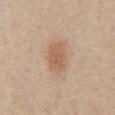Findings:
* biopsy status · total-body-photography surveillance lesion; no biopsy
* automated metrics · a lesion area of about 8.5 mm², an outline eccentricity of about 0.8 (0 = round, 1 = elongated), and two-axis asymmetry of about 0.2; a mean CIELAB color near L≈59 a*≈19 b*≈31, about 9 CIELAB-L* units darker than the surrounding skin, and a normalized lesion–skin contrast near 6.5; a nevus-likeness score of about 95/100
* patient · male, aged around 60
* diameter · ≈4 mm
* body site · the front of the torso
* image source · ~15 mm tile from a whole-body skin photo